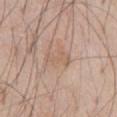diameter: about 3.5 mm | illumination: white-light illumination | anatomic site: the abdomen | patient: male, roughly 60 years of age | image source: 15 mm crop, total-body photography.